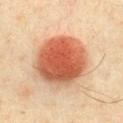Clinical impression: This lesion was catalogued during total-body skin photography and was not selected for biopsy. Context: From the chest. The patient is a male roughly 40 years of age. A close-up tile cropped from a whole-body skin photograph, about 15 mm across. Automated tile analysis of the lesion measured a lesion area of about 33 mm², an outline eccentricity of about 0.35 (0 = round, 1 = elongated), and a shape-asymmetry score of about 0.1 (0 = symmetric). Captured under cross-polarized illumination.